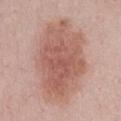notes — total-body-photography surveillance lesion; no biopsy | patient — female, about 50 years old | location — the front of the torso | image — total-body-photography crop, ~15 mm field of view.